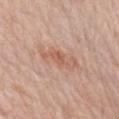Q: Is there a histopathology result?
A: imaged on a skin check; not biopsied
Q: Automated lesion metrics?
A: an area of roughly 8.5 mm², an eccentricity of roughly 0.9, and a shape-asymmetry score of about 0.35 (0 = symmetric); an automated nevus-likeness rating near 5 out of 100 and a detector confidence of about 100 out of 100 that the crop contains a lesion
Q: Where on the body is the lesion?
A: the right upper arm
Q: Lesion size?
A: about 5 mm
Q: How was the tile lit?
A: white-light illumination
Q: Who is the patient?
A: male, in their mid- to late 70s
Q: What is the imaging modality?
A: total-body-photography crop, ~15 mm field of view